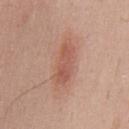Findings:
– biopsy status: no biopsy performed (imaged during a skin exam)
– body site: the mid back
– patient: male, aged around 35
– automated lesion analysis: an automated nevus-likeness rating near 70 out of 100 and lesion-presence confidence of about 100/100
– tile lighting: white-light
– image source: total-body-photography crop, ~15 mm field of view
– size: about 6 mm